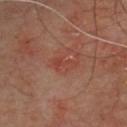No biopsy was performed on this lesion — it was imaged during a full skin examination and was not determined to be concerning. The total-body-photography lesion software estimated a mean CIELAB color near L≈42 a*≈26 b*≈28, roughly 5 lightness units darker than nearby skin, and a normalized lesion–skin contrast near 5. And it measured a within-lesion color-variation index near 3/10 and radial color variation of about 1. It also reported a nevus-likeness score of about 0/100 and a detector confidence of about 100 out of 100 that the crop contains a lesion. A male patient aged approximately 65. The lesion is located on the chest. A lesion tile, about 15 mm wide, cut from a 3D total-body photograph. Imaged with cross-polarized lighting.